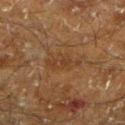Notes:
* follow-up — catalogued during a skin exam; not biopsied
* automated metrics — a footprint of about 6 mm², an outline eccentricity of about 0.9 (0 = round, 1 = elongated), and a shape-asymmetry score of about 0.4 (0 = symmetric); a border-irregularity index near 5.5/10, a color-variation rating of about 1.5/10, and peripheral color asymmetry of about 0.5; a classifier nevus-likeness of about 0/100 and a detector confidence of about 90 out of 100 that the crop contains a lesion
* body site — the leg
* lesion diameter — about 4.5 mm
* image source — total-body-photography crop, ~15 mm field of view
* subject — male, aged around 60